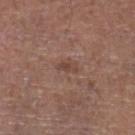Q: Was this lesion biopsied?
A: total-body-photography surveillance lesion; no biopsy
Q: How was the tile lit?
A: white-light illumination
Q: Where on the body is the lesion?
A: the left lower leg
Q: What kind of image is this?
A: 15 mm crop, total-body photography
Q: What are the patient's age and sex?
A: male, aged approximately 75
Q: What is the lesion's diameter?
A: about 2.5 mm